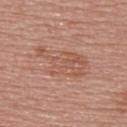Part of a total-body skin-imaging series; this lesion was reviewed on a skin check and was not flagged for biopsy. The recorded lesion diameter is about 7 mm. The subject is a female aged around 55. Imaged with white-light lighting. A region of skin cropped from a whole-body photographic capture, roughly 15 mm wide. From the back.